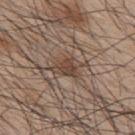A male patient aged 43–47.
Longest diameter approximately 4 mm.
The tile uses white-light illumination.
Cropped from a total-body skin-imaging series; the visible field is about 15 mm.
Automated image analysis of the tile measured a footprint of about 7.5 mm², a shape eccentricity near 0.75, and two-axis asymmetry of about 0.3. The software also gave an average lesion color of about L≈44 a*≈15 b*≈25 (CIELAB). The analysis additionally found a detector confidence of about 85 out of 100 that the crop contains a lesion.
The lesion is on the upper back.